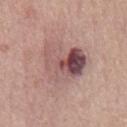The tile uses white-light illumination. Approximately 6.5 mm at its widest. A male patient, in their mid-70s. The lesion-visualizer software estimated a footprint of about 23 mm², a shape eccentricity near 0.6, and two-axis asymmetry of about 0.35. The software also gave a mean CIELAB color near L≈53 a*≈21 b*≈20, a lesion–skin lightness drop of about 12, and a normalized lesion–skin contrast near 8.5. A 15 mm close-up tile from a total-body photography series done for melanoma screening. From the chest.